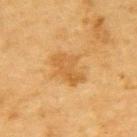follow-up: imaged on a skin check; not biopsied
image-analysis metrics: a lesion area of about 9 mm² and an outline eccentricity of about 0.75 (0 = round, 1 = elongated); border irregularity of about 4.5 on a 0–10 scale, a within-lesion color-variation index near 2.5/10, and radial color variation of about 1; a nevus-likeness score of about 5/100 and lesion-presence confidence of about 100/100
imaging modality: ~15 mm crop, total-body skin-cancer survey
lesion size: ~4 mm (longest diameter)
tile lighting: cross-polarized illumination
body site: the upper back
subject: male, aged 83 or over